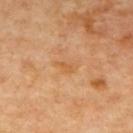biopsy status = total-body-photography surveillance lesion; no biopsy | subject = roughly 65 years of age | image source = 15 mm crop, total-body photography | site = the upper back.